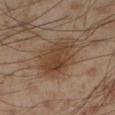Q: Was a biopsy performed?
A: catalogued during a skin exam; not biopsied
Q: What kind of image is this?
A: total-body-photography crop, ~15 mm field of view
Q: Who is the patient?
A: male, aged 53 to 57
Q: What is the anatomic site?
A: the leg
Q: Illumination type?
A: cross-polarized
Q: Automated lesion metrics?
A: border irregularity of about 2.5 on a 0–10 scale, a color-variation rating of about 4.5/10, and a peripheral color-asymmetry measure near 1.5; a detector confidence of about 100 out of 100 that the crop contains a lesion